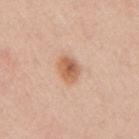| feature | finding |
|---|---|
| notes | catalogued during a skin exam; not biopsied |
| location | the right upper arm |
| TBP lesion metrics | a mean CIELAB color near L≈61 a*≈22 b*≈34, a lesion–skin lightness drop of about 12, and a normalized lesion–skin contrast near 8.5; a border-irregularity index near 1.5/10, a within-lesion color-variation index near 5/10, and peripheral color asymmetry of about 1.5; a nevus-likeness score of about 95/100 and lesion-presence confidence of about 100/100 |
| patient | female, aged approximately 40 |
| diameter | about 3 mm |
| image | total-body-photography crop, ~15 mm field of view |
| lighting | white-light |